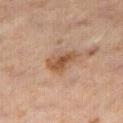biopsy status: catalogued during a skin exam; not biopsied | anatomic site: the right thigh | diameter: ≈4 mm | tile lighting: cross-polarized illumination | imaging modality: ~15 mm tile from a whole-body skin photo | patient: male, in their 60s.